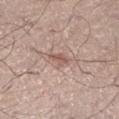Recorded during total-body skin imaging; not selected for excision or biopsy. The tile uses white-light illumination. The lesion's longest dimension is about 2.5 mm. A male patient, approximately 55 years of age. Cropped from a whole-body photographic skin survey; the tile spans about 15 mm.